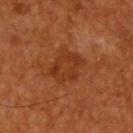This lesion was catalogued during total-body skin photography and was not selected for biopsy. A male subject, aged approximately 60. A 15 mm close-up extracted from a 3D total-body photography capture. About 4 mm across. The tile uses cross-polarized illumination. On the upper back.